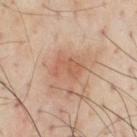<lesion>
<biopsy_status>not biopsied; imaged during a skin examination</biopsy_status>
<site>chest</site>
<patient>
  <sex>male</sex>
  <age_approx>55</age_approx>
</patient>
<image>
  <source>total-body photography crop</source>
  <field_of_view_mm>15</field_of_view_mm>
</image>
</lesion>